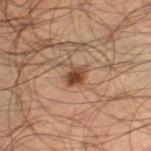notes: imaged on a skin check; not biopsied
patient: male, roughly 50 years of age
tile lighting: cross-polarized
diameter: about 2.5 mm
image: ~15 mm tile from a whole-body skin photo
anatomic site: the left thigh
TBP lesion metrics: an average lesion color of about L≈34 a*≈16 b*≈25 (CIELAB) and a lesion-to-skin contrast of about 9.5 (normalized; higher = more distinct)